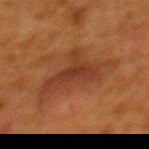{
  "biopsy_status": "not biopsied; imaged during a skin examination",
  "image": {
    "source": "total-body photography crop",
    "field_of_view_mm": 15
  },
  "patient": {
    "sex": "female",
    "age_approx": 50
  },
  "lighting": "cross-polarized",
  "lesion_size": {
    "long_diameter_mm_approx": 7.5
  },
  "site": "upper back",
  "automated_metrics": {
    "area_mm2_approx": 21.0,
    "eccentricity": 0.8,
    "cielab_L": 32,
    "cielab_a": 21,
    "cielab_b": 29,
    "vs_skin_darker_L": 7.0,
    "vs_skin_contrast_norm": 7.0,
    "nevus_likeness_0_100": 0,
    "lesion_detection_confidence_0_100": 100
  }
}